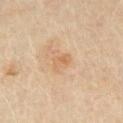{"lighting": "cross-polarized", "lesion_size": {"long_diameter_mm_approx": 2.5}, "image": {"source": "total-body photography crop", "field_of_view_mm": 15}, "patient": {"sex": "male", "age_approx": 60}, "automated_metrics": {"cielab_L": 57, "cielab_a": 17, "cielab_b": 33, "vs_skin_contrast_norm": 5.0, "border_irregularity_0_10": 2.5, "color_variation_0_10": 3.0, "peripheral_color_asymmetry": 1.0, "nevus_likeness_0_100": 0}, "site": "chest"}